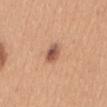Imaged during a routine full-body skin examination; the lesion was not biopsied and no histopathology is available.
The tile uses white-light illumination.
A female subject aged around 30.
Measured at roughly 3 mm in maximum diameter.
The lesion is located on the mid back.
A 15 mm crop from a total-body photograph taken for skin-cancer surveillance.